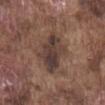The lesion is on the abdomen. Captured under white-light illumination. Automated tile analysis of the lesion measured an area of roughly 14 mm², an eccentricity of roughly 0.8, and a shape-asymmetry score of about 0.25 (0 = symmetric). The software also gave a mean CIELAB color near L≈38 a*≈15 b*≈20, roughly 10 lightness units darker than nearby skin, and a normalized border contrast of about 9.5. It also reported a border-irregularity rating of about 3.5/10, internal color variation of about 5.5 on a 0–10 scale, and a peripheral color-asymmetry measure near 2. Longest diameter approximately 6 mm. This image is a 15 mm lesion crop taken from a total-body photograph. A male patient, aged 73 to 77.